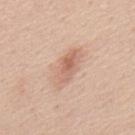follow-up: catalogued during a skin exam; not biopsied | TBP lesion metrics: about 9 CIELAB-L* units darker than the surrounding skin and a normalized border contrast of about 6; border irregularity of about 2.5 on a 0–10 scale and a color-variation rating of about 5.5/10 | lesion size: ~5 mm (longest diameter) | acquisition: ~15 mm crop, total-body skin-cancer survey | subject: male, aged 43 to 47 | body site: the mid back | illumination: white-light illumination.